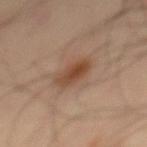biopsy status = no biopsy performed (imaged during a skin exam); TBP lesion metrics = border irregularity of about 2 on a 0–10 scale and a peripheral color-asymmetry measure near 1; lesion size = about 4 mm; subject = male, roughly 50 years of age; lighting = cross-polarized illumination; image = total-body-photography crop, ~15 mm field of view; anatomic site = the mid back.